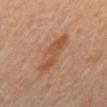Findings:
• follow-up: total-body-photography surveillance lesion; no biopsy
• diameter: ~5.5 mm (longest diameter)
• acquisition: 15 mm crop, total-body photography
• site: the mid back
• patient: male, in their mid-60s
• TBP lesion metrics: an area of roughly 7 mm²; a nevus-likeness score of about 60/100
• tile lighting: cross-polarized illumination